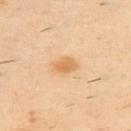Case summary:
- notes · imaged on a skin check; not biopsied
- lighting · cross-polarized illumination
- patient · male, aged approximately 40
- anatomic site · the back
- acquisition · ~15 mm crop, total-body skin-cancer survey